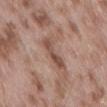Recorded during total-body skin imaging; not selected for excision or biopsy.
The tile uses white-light illumination.
Automated tile analysis of the lesion measured an average lesion color of about L≈50 a*≈20 b*≈26 (CIELAB), a lesion–skin lightness drop of about 10, and a lesion-to-skin contrast of about 7.5 (normalized; higher = more distinct). The software also gave border irregularity of about 5 on a 0–10 scale and a within-lesion color-variation index near 3/10. The analysis additionally found lesion-presence confidence of about 85/100.
From the lower back.
Cropped from a total-body skin-imaging series; the visible field is about 15 mm.
A male subject approximately 55 years of age.
The recorded lesion diameter is about 4.5 mm.